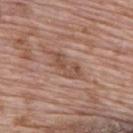Recorded during total-body skin imaging; not selected for excision or biopsy. A region of skin cropped from a whole-body photographic capture, roughly 15 mm wide. The lesion is on the back. A male patient about 70 years old.